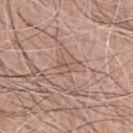On the chest.
The subject is a male aged approximately 60.
A 15 mm close-up extracted from a 3D total-body photography capture.